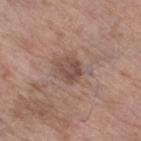Q: What is the imaging modality?
A: ~15 mm tile from a whole-body skin photo
Q: Where on the body is the lesion?
A: the left lower leg
Q: Patient demographics?
A: female, about 85 years old or older